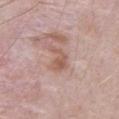follow-up = imaged on a skin check; not biopsied | patient = male, aged approximately 70 | imaging modality = ~15 mm crop, total-body skin-cancer survey | location = the abdomen.